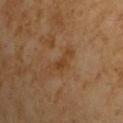Q: Was a biopsy performed?
A: imaged on a skin check; not biopsied
Q: What are the patient's age and sex?
A: male, aged approximately 65
Q: What kind of image is this?
A: 15 mm crop, total-body photography
Q: How was the tile lit?
A: cross-polarized
Q: Lesion size?
A: about 4 mm
Q: Automated lesion metrics?
A: a footprint of about 4.5 mm² and a shape-asymmetry score of about 0.55 (0 = symmetric); an average lesion color of about L≈40 a*≈19 b*≈34 (CIELAB), a lesion–skin lightness drop of about 6, and a lesion-to-skin contrast of about 6 (normalized; higher = more distinct); a border-irregularity rating of about 7/10, a color-variation rating of about 0/10, and peripheral color asymmetry of about 0